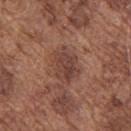Notes:
* follow-up · total-body-photography surveillance lesion; no biopsy
* diameter · ≈4.5 mm
* site · the upper back
* acquisition · ~15 mm tile from a whole-body skin photo
* subject · male, approximately 75 years of age
* tile lighting · white-light illumination